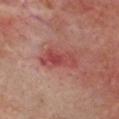A male subject aged around 65.
A 15 mm close-up tile from a total-body photography series done for melanoma screening.
Approximately 4 mm at its widest.
On the head or neck.
This is a cross-polarized tile.